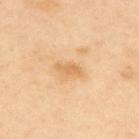Findings:
* biopsy status: catalogued during a skin exam; not biopsied
* image source: ~15 mm tile from a whole-body skin photo
* anatomic site: the upper back
* subject: female, roughly 40 years of age